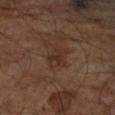| feature | finding |
|---|---|
| image source | ~15 mm tile from a whole-body skin photo |
| subject | male, about 65 years old |
| location | the right upper arm |